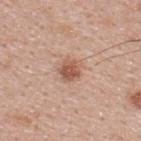Findings:
- notes: catalogued during a skin exam; not biopsied
- lesion size: ~2.5 mm (longest diameter)
- anatomic site: the upper back
- acquisition: 15 mm crop, total-body photography
- lighting: white-light illumination
- subject: male, about 40 years old